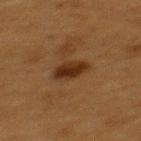This lesion was catalogued during total-body skin photography and was not selected for biopsy. A close-up tile cropped from a whole-body skin photograph, about 15 mm across. The lesion is located on the upper back. The subject is a female aged approximately 40.